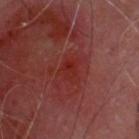Assessment: Captured during whole-body skin photography for melanoma surveillance; the lesion was not biopsied. Background: This is a cross-polarized tile. An algorithmic analysis of the crop reported a footprint of about 5 mm², an outline eccentricity of about 0.55 (0 = round, 1 = elongated), and a shape-asymmetry score of about 0.35 (0 = symmetric). And it measured a lesion color around L≈28 a*≈31 b*≈27 in CIELAB, a lesion–skin lightness drop of about 5, and a lesion-to-skin contrast of about 6.5 (normalized; higher = more distinct). The software also gave border irregularity of about 4 on a 0–10 scale and a within-lesion color-variation index near 4/10. And it measured a nevus-likeness score of about 0/100 and a detector confidence of about 100 out of 100 that the crop contains a lesion. A male subject about 60 years old. The recorded lesion diameter is about 2.5 mm. The lesion is located on the head or neck. This image is a 15 mm lesion crop taken from a total-body photograph.